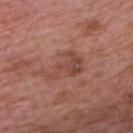The lesion was photographed on a routine skin check and not biopsied; there is no pathology result.
A male patient approximately 65 years of age.
This is a white-light tile.
A roughly 15 mm field-of-view crop from a total-body skin photograph.
Measured at roughly 5.5 mm in maximum diameter.
The lesion is located on the upper back.